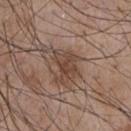This lesion was catalogued during total-body skin photography and was not selected for biopsy. A male patient, aged 53 to 57. On the chest. A lesion tile, about 15 mm wide, cut from a 3D total-body photograph.